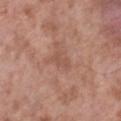Assessment:
The lesion was tiled from a total-body skin photograph and was not biopsied.
Image and clinical context:
The recorded lesion diameter is about 3 mm. A male subject about 55 years old. Imaged with white-light lighting. The lesion is on the leg. This image is a 15 mm lesion crop taken from a total-body photograph. Automated image analysis of the tile measured a footprint of about 3 mm² and a symmetry-axis asymmetry near 0.4. The analysis additionally found a border-irregularity index near 4.5/10, internal color variation of about 0 on a 0–10 scale, and a peripheral color-asymmetry measure near 0.